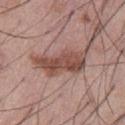Q: Is there a histopathology result?
A: catalogued during a skin exam; not biopsied
Q: What kind of image is this?
A: 15 mm crop, total-body photography
Q: What are the patient's age and sex?
A: male, in their mid-50s
Q: Where on the body is the lesion?
A: the abdomen
Q: How was the tile lit?
A: white-light
Q: What is the lesion's diameter?
A: ~6.5 mm (longest diameter)